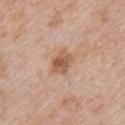workup: total-body-photography surveillance lesion; no biopsy
lesion size: ≈3.5 mm
automated lesion analysis: a mean CIELAB color near L≈57 a*≈21 b*≈32; a detector confidence of about 100 out of 100 that the crop contains a lesion
subject: female, roughly 70 years of age
body site: the chest
illumination: white-light illumination
imaging modality: 15 mm crop, total-body photography